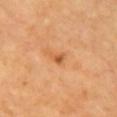No biopsy was performed on this lesion — it was imaged during a full skin examination and was not determined to be concerning. A region of skin cropped from a whole-body photographic capture, roughly 15 mm wide. Automated image analysis of the tile measured a footprint of about 2.5 mm², an outline eccentricity of about 0.7 (0 = round, 1 = elongated), and a symmetry-axis asymmetry near 0.4. The software also gave a mean CIELAB color near L≈60 a*≈28 b*≈44 and a normalized lesion–skin contrast near 6.5. And it measured lesion-presence confidence of about 100/100. From the chest. The recorded lesion diameter is about 2 mm. Captured under cross-polarized illumination. A female subject aged 68 to 72.